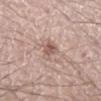Acquisition and patient details: A 15 mm close-up extracted from a 3D total-body photography capture. The patient is a male aged 38–42. About 3.5 mm across. An algorithmic analysis of the crop reported a lesion area of about 4.5 mm², an eccentricity of roughly 0.85, and a symmetry-axis asymmetry near 0.35. The analysis additionally found an average lesion color of about L≈57 a*≈18 b*≈25 (CIELAB), a lesion–skin lightness drop of about 10, and a lesion-to-skin contrast of about 7 (normalized; higher = more distinct). The software also gave border irregularity of about 3.5 on a 0–10 scale, internal color variation of about 3 on a 0–10 scale, and a peripheral color-asymmetry measure near 1. It also reported a nevus-likeness score of about 5/100 and a lesion-detection confidence of about 100/100. This is a white-light tile. Located on the right lower leg.